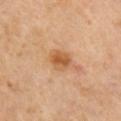Imaged during a routine full-body skin examination; the lesion was not biopsied and no histopathology is available. Cropped from a whole-body photographic skin survey; the tile spans about 15 mm. The recorded lesion diameter is about 2.5 mm. The total-body-photography lesion software estimated an area of roughly 5.5 mm², a shape eccentricity near 0.55, and two-axis asymmetry of about 0.2. It also reported a lesion–skin lightness drop of about 10. And it measured a border-irregularity rating of about 2/10, internal color variation of about 4.5 on a 0–10 scale, and a peripheral color-asymmetry measure near 1.5. On the right upper arm. A male subject, in their mid-50s.